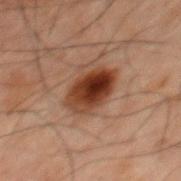biopsy status: no biopsy performed (imaged during a skin exam).